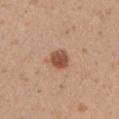Recorded during total-body skin imaging; not selected for excision or biopsy. A female subject about 30 years old. About 3 mm across. A lesion tile, about 15 mm wide, cut from a 3D total-body photograph. The lesion is located on the right upper arm. Captured under white-light illumination.